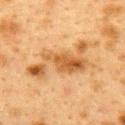• biopsy status — catalogued during a skin exam; not biopsied
• illumination — cross-polarized illumination
• image — ~15 mm tile from a whole-body skin photo
• body site — the upper back
• subject — female, aged around 40